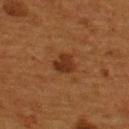Imaged during a routine full-body skin examination; the lesion was not biopsied and no histopathology is available.
Automated tile analysis of the lesion measured a shape eccentricity near 0.3 and a symmetry-axis asymmetry near 0.25. The analysis additionally found a lesion color around L≈30 a*≈22 b*≈31 in CIELAB and a lesion–skin lightness drop of about 9. The software also gave radial color variation of about 1.
This is a cross-polarized tile.
The lesion is on the upper back.
The subject is a male aged 53 to 57.
Cropped from a total-body skin-imaging series; the visible field is about 15 mm.
Measured at roughly 2.5 mm in maximum diameter.